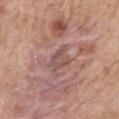This lesion was catalogued during total-body skin photography and was not selected for biopsy. The patient is a male aged 58 to 62. A 15 mm crop from a total-body photograph taken for skin-cancer surveillance. This is a white-light tile. The lesion is located on the chest. Automated image analysis of the tile measured an outline eccentricity of about 0.5 (0 = round, 1 = elongated) and a symmetry-axis asymmetry near 0.45. It also reported border irregularity of about 4.5 on a 0–10 scale, a within-lesion color-variation index near 3/10, and a peripheral color-asymmetry measure near 1. Longest diameter approximately 2.5 mm.